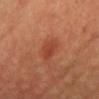notes: total-body-photography surveillance lesion; no biopsy
tile lighting: cross-polarized illumination
diameter: about 3.5 mm
location: the head or neck
image source: ~15 mm crop, total-body skin-cancer survey
automated metrics: a footprint of about 5 mm², an eccentricity of roughly 0.9, and a shape-asymmetry score of about 0.3 (0 = symmetric)
patient: female, aged around 40